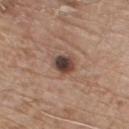Imaged during a routine full-body skin examination; the lesion was not biopsied and no histopathology is available. A region of skin cropped from a whole-body photographic capture, roughly 15 mm wide. Approximately 3 mm at its widest. The patient is a male in their mid- to late 70s. Captured under white-light illumination. From the chest.